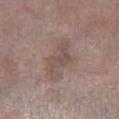Part of a total-body skin-imaging series; this lesion was reviewed on a skin check and was not flagged for biopsy.